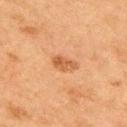{"biopsy_status": "not biopsied; imaged during a skin examination", "automated_metrics": {"area_mm2_approx": 4.0, "eccentricity": 0.85, "shape_asymmetry": 0.35, "border_irregularity_0_10": 3.0, "color_variation_0_10": 3.0, "peripheral_color_asymmetry": 1.0, "nevus_likeness_0_100": 85, "lesion_detection_confidence_0_100": 100}, "patient": {"sex": "male", "age_approx": 75}, "lighting": "cross-polarized", "lesion_size": {"long_diameter_mm_approx": 3.0}, "site": "upper back", "image": {"source": "total-body photography crop", "field_of_view_mm": 15}}